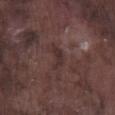{
  "lesion_size": {
    "long_diameter_mm_approx": 3.0
  },
  "lighting": "white-light",
  "automated_metrics": {
    "eccentricity": 0.85,
    "shape_asymmetry": 0.55,
    "border_irregularity_0_10": 5.5,
    "color_variation_0_10": 0.0
  },
  "image": {
    "source": "total-body photography crop",
    "field_of_view_mm": 15
  },
  "site": "right lower leg",
  "patient": {
    "sex": "male",
    "age_approx": 75
  }
}